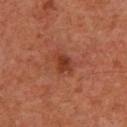follow-up: total-body-photography surveillance lesion; no biopsy | lesion size: ≈2.5 mm | subject: male, in their 60s | image-analysis metrics: a lesion area of about 4.5 mm², an outline eccentricity of about 0.6 (0 = round, 1 = elongated), and a shape-asymmetry score of about 0.25 (0 = symmetric); a lesion color around L≈34 a*≈26 b*≈30 in CIELAB, a lesion–skin lightness drop of about 8, and a normalized lesion–skin contrast near 7; a border-irregularity rating of about 2.5/10, a color-variation rating of about 2.5/10, and a peripheral color-asymmetry measure near 0.5; lesion-presence confidence of about 100/100 | image: ~15 mm tile from a whole-body skin photo | location: the chest | tile lighting: cross-polarized illumination.